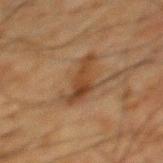Clinical impression:
Imaged during a routine full-body skin examination; the lesion was not biopsied and no histopathology is available.
Background:
On the upper back. The tile uses cross-polarized illumination. The recorded lesion diameter is about 5 mm. A male patient in their mid- to late 60s. A 15 mm close-up extracted from a 3D total-body photography capture.